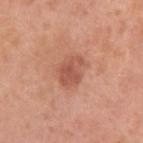No biopsy was performed on this lesion — it was imaged during a full skin examination and was not determined to be concerning. Approximately 3 mm at its widest. The lesion is located on the left upper arm. The total-body-photography lesion software estimated border irregularity of about 3.5 on a 0–10 scale, internal color variation of about 2.5 on a 0–10 scale, and radial color variation of about 0.5. A female patient, approximately 40 years of age. Cropped from a whole-body photographic skin survey; the tile spans about 15 mm.